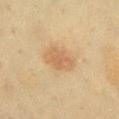<tbp_lesion>
<biopsy_status>not biopsied; imaged during a skin examination</biopsy_status>
<lesion_size>
  <long_diameter_mm_approx>4.5</long_diameter_mm_approx>
</lesion_size>
<image>
  <source>total-body photography crop</source>
  <field_of_view_mm>15</field_of_view_mm>
</image>
<patient>
  <sex>female</sex>
  <age_approx>60</age_approx>
</patient>
<site>chest</site>
<lighting>cross-polarized</lighting>
</tbp_lesion>